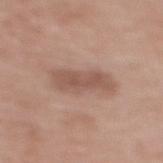Impression: The lesion was tiled from a total-body skin photograph and was not biopsied.